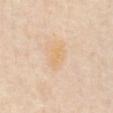Acquisition and patient details: Automated tile analysis of the lesion measured an outline eccentricity of about 0.8 (0 = round, 1 = elongated). And it measured a lesion color around L≈72 a*≈15 b*≈39 in CIELAB, about 4 CIELAB-L* units darker than the surrounding skin, and a normalized lesion–skin contrast near 6. The analysis additionally found a border-irregularity rating of about 4/10. The software also gave a nevus-likeness score of about 0/100 and lesion-presence confidence of about 100/100. Measured at roughly 3 mm in maximum diameter. On the abdomen. The patient is a male in their mid- to late 60s. Imaged with cross-polarized lighting. Cropped from a whole-body photographic skin survey; the tile spans about 15 mm.